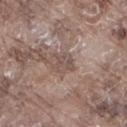The lesion was tiled from a total-body skin photograph and was not biopsied.
Imaged with white-light lighting.
Located on the right thigh.
Automated tile analysis of the lesion measured a footprint of about 5 mm², an outline eccentricity of about 0.5 (0 = round, 1 = elongated), and two-axis asymmetry of about 0.35. And it measured a mean CIELAB color near L≈50 a*≈16 b*≈22, roughly 8 lightness units darker than nearby skin, and a lesion-to-skin contrast of about 6 (normalized; higher = more distinct). It also reported a border-irregularity index near 4/10 and a color-variation rating of about 4/10. The software also gave a classifier nevus-likeness of about 0/100 and lesion-presence confidence of about 50/100.
The patient is a male about 75 years old.
A 15 mm crop from a total-body photograph taken for skin-cancer surveillance.
Measured at roughly 3 mm in maximum diameter.